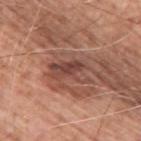Q: Is there a histopathology result?
A: no biopsy performed (imaged during a skin exam)
Q: What is the lesion's diameter?
A: about 4.5 mm
Q: Where on the body is the lesion?
A: the upper back
Q: What did automated image analysis measure?
A: an area of roughly 9.5 mm², an outline eccentricity of about 0.85 (0 = round, 1 = elongated), and a shape-asymmetry score of about 0.3 (0 = symmetric); a border-irregularity rating of about 4.5/10, a color-variation rating of about 6/10, and a peripheral color-asymmetry measure near 2.5
Q: Who is the patient?
A: male, aged 58 to 62
Q: How was this image acquired?
A: ~15 mm crop, total-body skin-cancer survey
Q: Illumination type?
A: white-light